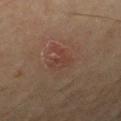{"biopsy_status": "not biopsied; imaged during a skin examination", "patient": {"sex": "male", "age_approx": 70}, "image": {"source": "total-body photography crop", "field_of_view_mm": 15}, "site": "right lower leg"}